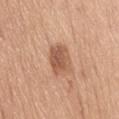The lesion was tiled from a total-body skin photograph and was not biopsied. The patient is a female in their mid- to late 60s. Captured under white-light illumination. From the front of the torso. Approximately 3.5 mm at its widest. Cropped from a whole-body photographic skin survey; the tile spans about 15 mm.